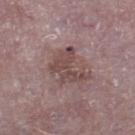Impression:
Imaged during a routine full-body skin examination; the lesion was not biopsied and no histopathology is available.
Context:
A male patient in their mid-70s. This image is a 15 mm lesion crop taken from a total-body photograph. The lesion is located on the leg.